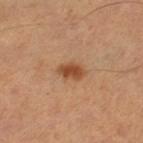Notes:
– workup: total-body-photography surveillance lesion; no biopsy
– subject: male, roughly 55 years of age
– illumination: cross-polarized illumination
– location: the left thigh
– image-analysis metrics: an area of roughly 4.5 mm² and a symmetry-axis asymmetry near 0.2; a mean CIELAB color near L≈46 a*≈23 b*≈34, roughly 11 lightness units darker than nearby skin, and a normalized lesion–skin contrast near 9; a nevus-likeness score of about 95/100
– diameter: ~3 mm (longest diameter)
– acquisition: total-body-photography crop, ~15 mm field of view